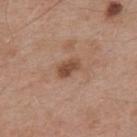Case summary:
* workup: imaged on a skin check; not biopsied
* tile lighting: white-light illumination
* size: ~3 mm (longest diameter)
* TBP lesion metrics: a lesion–skin lightness drop of about 12; a border-irregularity rating of about 2.5/10, internal color variation of about 2.5 on a 0–10 scale, and radial color variation of about 1
* image source: 15 mm crop, total-body photography
* anatomic site: the upper back
* subject: male, about 55 years old